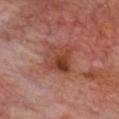Background: The lesion-visualizer software estimated an area of roughly 10 mm², a shape eccentricity near 0.7, and two-axis asymmetry of about 0.25. Located on the head or neck. This is a cross-polarized tile. The patient is a male roughly 65 years of age. A region of skin cropped from a whole-body photographic capture, roughly 15 mm wide.